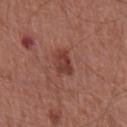Part of a total-body skin-imaging series; this lesion was reviewed on a skin check and was not flagged for biopsy.
A 15 mm crop from a total-body photograph taken for skin-cancer surveillance.
From the chest.
An algorithmic analysis of the crop reported an outline eccentricity of about 0.7 (0 = round, 1 = elongated) and a symmetry-axis asymmetry near 0.3. The analysis additionally found a lesion color around L≈40 a*≈25 b*≈26 in CIELAB, a lesion–skin lightness drop of about 9, and a normalized lesion–skin contrast near 7.5. And it measured a border-irregularity index near 2.5/10, a within-lesion color-variation index near 2.5/10, and radial color variation of about 1. The analysis additionally found a nevus-likeness score of about 65/100 and lesion-presence confidence of about 100/100.
The patient is a male aged around 65.
Measured at roughly 3 mm in maximum diameter.